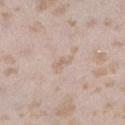biopsy status: total-body-photography surveillance lesion; no biopsy
imaging modality: 15 mm crop, total-body photography
tile lighting: white-light illumination
subject: female, about 25 years old
automated lesion analysis: a lesion area of about 2.5 mm²; an average lesion color of about L≈65 a*≈15 b*≈26 (CIELAB), a lesion–skin lightness drop of about 6, and a lesion-to-skin contrast of about 5 (normalized; higher = more distinct); border irregularity of about 4.5 on a 0–10 scale and internal color variation of about 0 on a 0–10 scale; a classifier nevus-likeness of about 0/100 and a detector confidence of about 95 out of 100 that the crop contains a lesion
location: the left lower leg
size: ≈2.5 mm